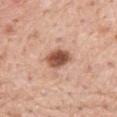{"biopsy_status": "not biopsied; imaged during a skin examination", "site": "back", "patient": {"sex": "male", "age_approx": 60}, "image": {"source": "total-body photography crop", "field_of_view_mm": 15}}